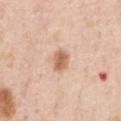<lesion>
  <biopsy_status>not biopsied; imaged during a skin examination</biopsy_status>
  <automated_metrics>
    <border_irregularity_0_10>1.5</border_irregularity_0_10>
    <color_variation_0_10>2.5</color_variation_0_10>
    <peripheral_color_asymmetry>1.0</peripheral_color_asymmetry>
    <nevus_likeness_0_100>90</nevus_likeness_0_100>
  </automated_metrics>
  <lesion_size>
    <long_diameter_mm_approx>2.5</long_diameter_mm_approx>
  </lesion_size>
  <lighting>white-light</lighting>
  <patient>
    <sex>male</sex>
    <age_approx>50</age_approx>
  </patient>
  <site>front of the torso</site>
  <image>
    <source>total-body photography crop</source>
    <field_of_view_mm>15</field_of_view_mm>
  </image>
</lesion>